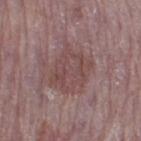Assessment:
Captured during whole-body skin photography for melanoma surveillance; the lesion was not biopsied.
Context:
The recorded lesion diameter is about 5 mm. A 15 mm close-up tile from a total-body photography series done for melanoma screening. This is a white-light tile. Located on the right thigh. The patient is a male aged 73–77.